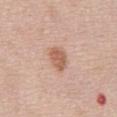Recorded during total-body skin imaging; not selected for excision or biopsy. Longest diameter approximately 3 mm. The lesion is on the abdomen. A male subject approximately 70 years of age. A 15 mm close-up tile from a total-body photography series done for melanoma screening. Captured under white-light illumination.